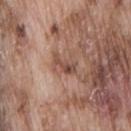Impression:
Part of a total-body skin-imaging series; this lesion was reviewed on a skin check and was not flagged for biopsy.
Acquisition and patient details:
Approximately 3 mm at its widest. The lesion is on the lower back. The total-body-photography lesion software estimated about 10 CIELAB-L* units darker than the surrounding skin and a normalized border contrast of about 7.5. And it measured a border-irregularity index near 6/10, a within-lesion color-variation index near 2/10, and a peripheral color-asymmetry measure near 0. The software also gave a classifier nevus-likeness of about 0/100 and a lesion-detection confidence of about 100/100. Captured under white-light illumination. A male subject aged 73 to 77. Cropped from a whole-body photographic skin survey; the tile spans about 15 mm.